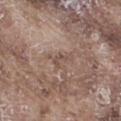Clinical impression:
This lesion was catalogued during total-body skin photography and was not selected for biopsy.
Context:
Longest diameter approximately 2.5 mm. Automated image analysis of the tile measured a mean CIELAB color near L≈50 a*≈16 b*≈23, about 7 CIELAB-L* units darker than the surrounding skin, and a lesion-to-skin contrast of about 5 (normalized; higher = more distinct). The analysis additionally found a border-irregularity index near 6/10, a color-variation rating of about 0/10, and peripheral color asymmetry of about 0. It also reported a classifier nevus-likeness of about 0/100 and lesion-presence confidence of about 70/100. A lesion tile, about 15 mm wide, cut from a 3D total-body photograph. A male subject, aged 73–77. Located on the right thigh.